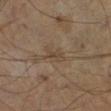biopsy status — no biopsy performed (imaged during a skin exam) | size — ~2.5 mm (longest diameter) | automated metrics — a lesion area of about 3 mm², an eccentricity of roughly 0.9, and two-axis asymmetry of about 0.35; a lesion color around L≈41 a*≈12 b*≈26 in CIELAB, about 6 CIELAB-L* units darker than the surrounding skin, and a normalized lesion–skin contrast near 5 | location — the leg | subject — male, roughly 60 years of age | image source — ~15 mm tile from a whole-body skin photo | illumination — cross-polarized illumination.